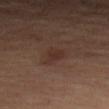Impression: Captured during whole-body skin photography for melanoma surveillance; the lesion was not biopsied. Background: The subject is a female aged 63–67. Automated image analysis of the tile measured a color-variation rating of about 2/10 and radial color variation of about 1. The software also gave a classifier nevus-likeness of about 45/100 and a lesion-detection confidence of about 100/100. Measured at roughly 3 mm in maximum diameter. A 15 mm close-up extracted from a 3D total-body photography capture. On the left forearm. Imaged with cross-polarized lighting.